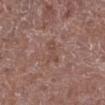Assessment: The lesion was tiled from a total-body skin photograph and was not biopsied.